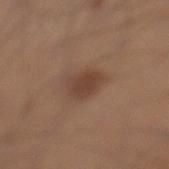| feature | finding |
|---|---|
| follow-up | imaged on a skin check; not biopsied |
| TBP lesion metrics | a footprint of about 7 mm², an outline eccentricity of about 0.75 (0 = round, 1 = elongated), and two-axis asymmetry of about 0.2; peripheral color asymmetry of about 0.5 |
| location | the left lower leg |
| diameter | about 3.5 mm |
| imaging modality | ~15 mm crop, total-body skin-cancer survey |
| subject | male, approximately 30 years of age |
| tile lighting | white-light |